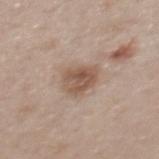{
  "biopsy_status": "not biopsied; imaged during a skin examination",
  "image": {
    "source": "total-body photography crop",
    "field_of_view_mm": 15
  },
  "patient": {
    "sex": "female",
    "age_approx": 40
  },
  "lighting": "white-light",
  "automated_metrics": {
    "area_mm2_approx": 8.5,
    "eccentricity": 0.55,
    "lesion_detection_confidence_0_100": 100
  },
  "site": "mid back",
  "lesion_size": {
    "long_diameter_mm_approx": 3.5
  }
}